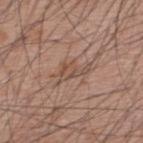A close-up tile cropped from a whole-body skin photograph, about 15 mm across. The patient is a male aged 58 to 62. From the back. Measured at roughly 4 mm in maximum diameter. Captured under white-light illumination. Automated image analysis of the tile measured a lesion–skin lightness drop of about 7 and a normalized border contrast of about 5.5. The software also gave a border-irregularity rating of about 6/10, internal color variation of about 3 on a 0–10 scale, and radial color variation of about 1.